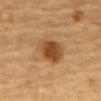No biopsy was performed on this lesion — it was imaged during a full skin examination and was not determined to be concerning.